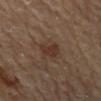notes: total-body-photography surveillance lesion; no biopsy | patient: male, aged around 85 or older | tile lighting: cross-polarized | lesion diameter: about 3 mm | body site: the back | acquisition: total-body-photography crop, ~15 mm field of view.